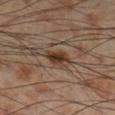Assessment: Captured during whole-body skin photography for melanoma surveillance; the lesion was not biopsied. Clinical summary: A male patient, in their mid-50s. A roughly 15 mm field-of-view crop from a total-body skin photograph. About 3 mm across. This is a cross-polarized tile. The lesion is located on the right lower leg.